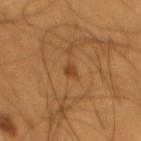Q: Automated lesion metrics?
A: a footprint of about 2 mm², a shape eccentricity near 0.65, and two-axis asymmetry of about 0.3; an average lesion color of about L≈40 a*≈22 b*≈36 (CIELAB) and a normalized lesion–skin contrast near 7; radial color variation of about 0; a detector confidence of about 100 out of 100 that the crop contains a lesion
Q: Where on the body is the lesion?
A: the mid back
Q: Lesion size?
A: ≈1.5 mm
Q: Patient demographics?
A: male, aged 58–62
Q: How was this image acquired?
A: total-body-photography crop, ~15 mm field of view